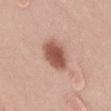Assessment:
The lesion was tiled from a total-body skin photograph and was not biopsied.
Clinical summary:
Cropped from a whole-body photographic skin survey; the tile spans about 15 mm. A male subject aged 48 to 52. The recorded lesion diameter is about 4.5 mm. Imaged with white-light lighting. Located on the chest.